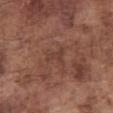{"biopsy_status": "not biopsied; imaged during a skin examination", "image": {"source": "total-body photography crop", "field_of_view_mm": 15}, "site": "abdomen", "automated_metrics": {"area_mm2_approx": 4.0, "eccentricity": 0.75, "shape_asymmetry": 0.55, "border_irregularity_0_10": 6.0, "color_variation_0_10": 0.5, "peripheral_color_asymmetry": 0.0, "nevus_likeness_0_100": 0, "lesion_detection_confidence_0_100": 95}, "patient": {"sex": "male", "age_approx": 75}, "lighting": "white-light"}